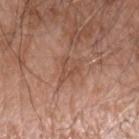biopsy_status: not biopsied; imaged during a skin examination
automated_metrics:
  cielab_L: 49
  cielab_a: 22
  cielab_b: 29
  vs_skin_contrast_norm: 5.5
  border_irregularity_0_10: 8.0
  color_variation_0_10: 0.0
  peripheral_color_asymmetry: 0.0
site: left forearm
lesion_size:
  long_diameter_mm_approx: 3.5
lighting: white-light
patient:
  sex: male
  age_approx: 60
image:
  source: total-body photography crop
  field_of_view_mm: 15